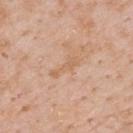Q: Is there a histopathology result?
A: no biopsy performed (imaged during a skin exam)
Q: What are the patient's age and sex?
A: male, about 60 years old
Q: Where on the body is the lesion?
A: the upper back
Q: What kind of image is this?
A: ~15 mm crop, total-body skin-cancer survey